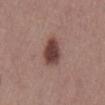This lesion was catalogued during total-body skin photography and was not selected for biopsy. Located on the abdomen. A 15 mm close-up tile from a total-body photography series done for melanoma screening. Imaged with white-light lighting. The recorded lesion diameter is about 4 mm. A male patient, approximately 40 years of age.